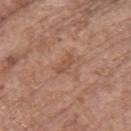biopsy status — imaged on a skin check; not biopsied
diameter — about 2.5 mm
tile lighting — white-light
subject — female, aged approximately 65
image — ~15 mm crop, total-body skin-cancer survey
location — the upper back